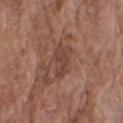Captured during whole-body skin photography for melanoma surveillance; the lesion was not biopsied. The patient is a male aged around 75. An algorithmic analysis of the crop reported an area of roughly 5.5 mm², an outline eccentricity of about 0.9 (0 = round, 1 = elongated), and a symmetry-axis asymmetry near 0.4. The software also gave a border-irregularity rating of about 5/10, a color-variation rating of about 1.5/10, and peripheral color asymmetry of about 0.5. Longest diameter approximately 4 mm. A lesion tile, about 15 mm wide, cut from a 3D total-body photograph. From the right upper arm.